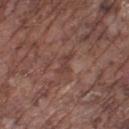Assessment:
This lesion was catalogued during total-body skin photography and was not selected for biopsy.
Clinical summary:
The lesion is located on the right lower leg. A lesion tile, about 15 mm wide, cut from a 3D total-body photograph. About 2.5 mm across. A male subject aged around 75. Automated image analysis of the tile measured an average lesion color of about L≈40 a*≈20 b*≈22 (CIELAB). And it measured border irregularity of about 4 on a 0–10 scale, a within-lesion color-variation index near 0/10, and a peripheral color-asymmetry measure near 0. The analysis additionally found a nevus-likeness score of about 0/100 and a detector confidence of about 55 out of 100 that the crop contains a lesion.